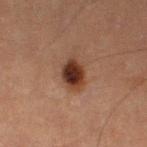Imaged during a routine full-body skin examination; the lesion was not biopsied and no histopathology is available.